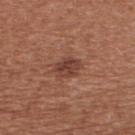Findings:
– biopsy status: imaged on a skin check; not biopsied
– imaging modality: ~15 mm tile from a whole-body skin photo
– location: the upper back
– patient: male, about 65 years old
– size: ≈3.5 mm
– lighting: white-light illumination